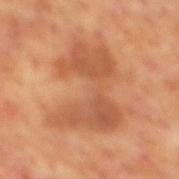This lesion was catalogued during total-body skin photography and was not selected for biopsy. Longest diameter approximately 7.5 mm. Cropped from a total-body skin-imaging series; the visible field is about 15 mm. A male subject, aged 68 to 72. Located on the mid back. Automated image analysis of the tile measured a border-irregularity rating of about 8/10 and a within-lesion color-variation index near 4/10. It also reported an automated nevus-likeness rating near 0 out of 100 and a lesion-detection confidence of about 100/100. The tile uses cross-polarized illumination.